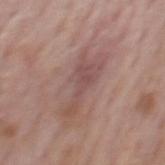follow-up — catalogued during a skin exam; not biopsied
lesion diameter — ~6 mm (longest diameter)
automated metrics — border irregularity of about 8 on a 0–10 scale, a color-variation rating of about 2.5/10, and a peripheral color-asymmetry measure near 0.5
site — the mid back
lighting — white-light
acquisition — 15 mm crop, total-body photography
patient — male, in their mid-70s